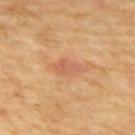The lesion was tiled from a total-body skin photograph and was not biopsied. A close-up tile cropped from a whole-body skin photograph, about 15 mm across. A male subject, about 70 years old. Longest diameter approximately 3.5 mm. From the upper back.